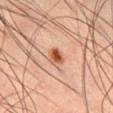| key | value |
|---|---|
| notes | no biopsy performed (imaged during a skin exam) |
| patient | male, aged approximately 45 |
| lesion diameter | ≈2.5 mm |
| imaging modality | 15 mm crop, total-body photography |
| image-analysis metrics | an area of roughly 4 mm², an outline eccentricity of about 0.4 (0 = round, 1 = elongated), and a symmetry-axis asymmetry near 0.25; a border-irregularity index near 2/10, internal color variation of about 4.5 on a 0–10 scale, and a peripheral color-asymmetry measure near 1.5 |
| tile lighting | cross-polarized |
| site | the chest |